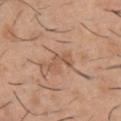Acquisition and patient details: Imaged with white-light lighting. A 15 mm close-up extracted from a 3D total-body photography capture. A male patient aged 38 to 42. The lesion is located on the chest. About 3 mm across.